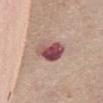Impression: Captured during whole-body skin photography for melanoma surveillance; the lesion was not biopsied. Background: The patient is a female approximately 70 years of age. Longest diameter approximately 4 mm. Cropped from a total-body skin-imaging series; the visible field is about 15 mm. The lesion is on the chest.